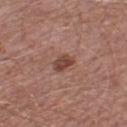Notes:
– location · the leg
– lesion diameter · about 3 mm
– subject · male, roughly 55 years of age
– automated metrics · an outline eccentricity of about 0.85 (0 = round, 1 = elongated) and a shape-asymmetry score of about 0.35 (0 = symmetric); a lesion color around L≈43 a*≈22 b*≈24 in CIELAB, a lesion–skin lightness drop of about 11, and a lesion-to-skin contrast of about 9 (normalized; higher = more distinct); border irregularity of about 3 on a 0–10 scale, a color-variation rating of about 2/10, and peripheral color asymmetry of about 0.5
– imaging modality · ~15 mm tile from a whole-body skin photo